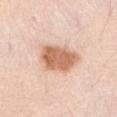workup: catalogued during a skin exam; not biopsied | location: the abdomen | size: ~5.5 mm (longest diameter) | patient: female, roughly 40 years of age | tile lighting: white-light illumination | image-analysis metrics: an average lesion color of about L≈65 a*≈22 b*≈33 (CIELAB), about 15 CIELAB-L* units darker than the surrounding skin, and a lesion-to-skin contrast of about 9.5 (normalized; higher = more distinct) | imaging modality: 15 mm crop, total-body photography.